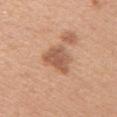Impression: The lesion was photographed on a routine skin check and not biopsied; there is no pathology result. Image and clinical context: This is a white-light tile. The lesion's longest dimension is about 4 mm. A female patient, aged 43–47. On the right upper arm. A lesion tile, about 15 mm wide, cut from a 3D total-body photograph.